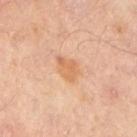Case summary:
– notes · total-body-photography surveillance lesion; no biopsy
– subject · in their mid-50s
– body site · the arm
– size · about 3 mm
– imaging modality · ~15 mm crop, total-body skin-cancer survey
– TBP lesion metrics · an automated nevus-likeness rating near 10 out of 100
– lighting · cross-polarized